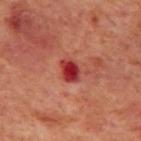workup=no biopsy performed (imaged during a skin exam) | patient=male, aged approximately 70 | anatomic site=the mid back | lighting=cross-polarized | lesion diameter=about 2.5 mm | image=~15 mm tile from a whole-body skin photo.